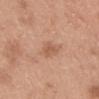Clinical impression: Part of a total-body skin-imaging series; this lesion was reviewed on a skin check and was not flagged for biopsy.